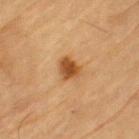notes = imaged on a skin check; not biopsied
illumination = cross-polarized illumination
acquisition = total-body-photography crop, ~15 mm field of view
TBP lesion metrics = border irregularity of about 2.5 on a 0–10 scale, a color-variation rating of about 4/10, and a peripheral color-asymmetry measure near 1
subject = male, in their mid-80s or older
anatomic site = the left upper arm